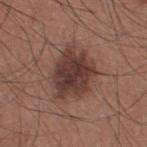Assessment:
The lesion was tiled from a total-body skin photograph and was not biopsied.
Acquisition and patient details:
About 6 mm across. A male subject aged approximately 35. Automated tile analysis of the lesion measured an area of roughly 20 mm², an eccentricity of roughly 0.6, and two-axis asymmetry of about 0.25. The tile uses white-light illumination. Cropped from a whole-body photographic skin survey; the tile spans about 15 mm. The lesion is on the upper back.